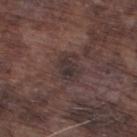{
  "biopsy_status": "not biopsied; imaged during a skin examination",
  "patient": {
    "sex": "male",
    "age_approx": 75
  },
  "lesion_size": {
    "long_diameter_mm_approx": 3.0
  },
  "automated_metrics": {
    "eccentricity": 0.75,
    "shape_asymmetry": 0.25,
    "lesion_detection_confidence_0_100": 95
  },
  "site": "left lower leg",
  "image": {
    "source": "total-body photography crop",
    "field_of_view_mm": 15
  }
}